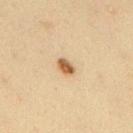Assessment:
The lesion was photographed on a routine skin check and not biopsied; there is no pathology result.
Context:
The recorded lesion diameter is about 2.5 mm. A 15 mm crop from a total-body photograph taken for skin-cancer surveillance. Captured under cross-polarized illumination. The lesion is on the back. A male patient aged 33–37.